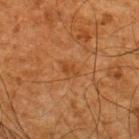<lesion>
<biopsy_status>not biopsied; imaged during a skin examination</biopsy_status>
<site>upper back</site>
<lighting>cross-polarized</lighting>
<lesion_size>
  <long_diameter_mm_approx>2.5</long_diameter_mm_approx>
</lesion_size>
<image>
  <source>total-body photography crop</source>
  <field_of_view_mm>15</field_of_view_mm>
</image>
<patient>
  <sex>male</sex>
  <age_approx>65</age_approx>
</patient>
</lesion>